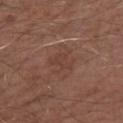Assessment:
Imaged during a routine full-body skin examination; the lesion was not biopsied and no histopathology is available.
Acquisition and patient details:
The subject is a male in their mid-50s. Automated tile analysis of the lesion measured an average lesion color of about L≈40 a*≈20 b*≈24 (CIELAB), roughly 5 lightness units darker than nearby skin, and a normalized border contrast of about 4.5. It also reported a nevus-likeness score of about 0/100 and a detector confidence of about 100 out of 100 that the crop contains a lesion. This is a white-light tile. Measured at roughly 3 mm in maximum diameter. Cropped from a total-body skin-imaging series; the visible field is about 15 mm. From the left forearm.